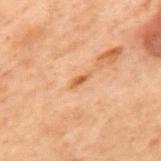Clinical impression: Part of a total-body skin-imaging series; this lesion was reviewed on a skin check and was not flagged for biopsy. Context: Longest diameter approximately 2.5 mm. A male patient roughly 70 years of age. This is a cross-polarized tile. A 15 mm close-up tile from a total-body photography series done for melanoma screening. The lesion is on the mid back.